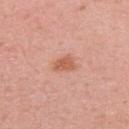The patient is a female about 35 years old.
About 3 mm across.
A close-up tile cropped from a whole-body skin photograph, about 15 mm across.
This is a white-light tile.
The lesion is on the right upper arm.